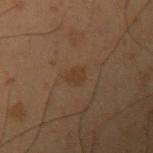biopsy status = total-body-photography surveillance lesion; no biopsy | patient = male, in their mid-50s | location = the left upper arm | illumination = cross-polarized illumination | image = total-body-photography crop, ~15 mm field of view | lesion diameter = ≈2.5 mm.